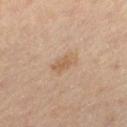{"biopsy_status": "not biopsied; imaged during a skin examination", "site": "left thigh", "image": {"source": "total-body photography crop", "field_of_view_mm": 15}, "lesion_size": {"long_diameter_mm_approx": 3.0}, "automated_metrics": {"cielab_L": 59, "cielab_a": 17, "cielab_b": 33, "vs_skin_darker_L": 8.0, "vs_skin_contrast_norm": 6.0, "nevus_likeness_0_100": 5, "lesion_detection_confidence_0_100": 100}, "lighting": "cross-polarized", "patient": {"sex": "male", "age_approx": 65}}